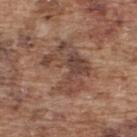– biopsy status — no biopsy performed (imaged during a skin exam)
– body site — the upper back
– imaging modality — ~15 mm tile from a whole-body skin photo
– tile lighting — white-light illumination
– diameter — about 6 mm
– patient — male, in their mid-70s